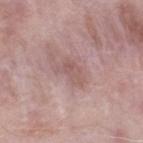{"biopsy_status": "not biopsied; imaged during a skin examination", "site": "leg", "lighting": "white-light", "image": {"source": "total-body photography crop", "field_of_view_mm": 15}, "patient": {"sex": "male", "age_approx": 40}, "automated_metrics": {"area_mm2_approx": 6.0, "eccentricity": 0.85, "shape_asymmetry": 0.5, "cielab_L": 56, "cielab_a": 20, "cielab_b": 21, "vs_skin_darker_L": 7.0, "vs_skin_contrast_norm": 5.0, "border_irregularity_0_10": 5.0, "color_variation_0_10": 3.0, "peripheral_color_asymmetry": 1.0}, "lesion_size": {"long_diameter_mm_approx": 3.5}}